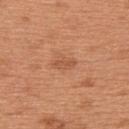The lesion was tiled from a total-body skin photograph and was not biopsied.
The tile uses white-light illumination.
Cropped from a total-body skin-imaging series; the visible field is about 15 mm.
From the upper back.
A male subject, about 65 years old.